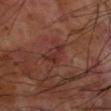Q: Was this lesion biopsied?
A: catalogued during a skin exam; not biopsied
Q: Automated lesion metrics?
A: a mean CIELAB color near L≈30 a*≈24 b*≈23, roughly 6 lightness units darker than nearby skin, and a normalized lesion–skin contrast near 6
Q: Patient demographics?
A: male, approximately 70 years of age
Q: What is the anatomic site?
A: the left forearm
Q: What kind of image is this?
A: 15 mm crop, total-body photography